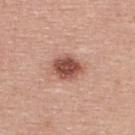{
  "biopsy_status": "not biopsied; imaged during a skin examination",
  "patient": {
    "sex": "female",
    "age_approx": 40
  },
  "lighting": "white-light",
  "image": {
    "source": "total-body photography crop",
    "field_of_view_mm": 15
  },
  "site": "back",
  "automated_metrics": {
    "area_mm2_approx": 9.5,
    "eccentricity": 0.65,
    "shape_asymmetry": 0.15,
    "nevus_likeness_0_100": 95,
    "lesion_detection_confidence_0_100": 100
  }
}